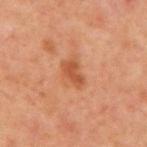Part of a total-body skin-imaging series; this lesion was reviewed on a skin check and was not flagged for biopsy. Automated tile analysis of the lesion measured a color-variation rating of about 1.5/10 and peripheral color asymmetry of about 0.5. The subject is a male aged 58–62. Located on the front of the torso. A close-up tile cropped from a whole-body skin photograph, about 15 mm across.